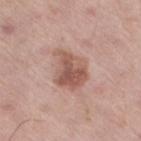Case summary:
• follow-up — no biopsy performed (imaged during a skin exam)
• size — about 4 mm
• site — the right thigh
• subject — male, aged 68 to 72
• imaging modality — total-body-photography crop, ~15 mm field of view
• illumination — white-light